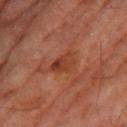The lesion was tiled from a total-body skin photograph and was not biopsied. A male subject aged approximately 70. Cropped from a total-body skin-imaging series; the visible field is about 15 mm. The lesion's longest dimension is about 4 mm. The lesion is on the right thigh. This is a cross-polarized tile.